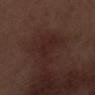The lesion was photographed on a routine skin check and not biopsied; there is no pathology result.
The tile uses white-light illumination.
Automated tile analysis of the lesion measured an area of roughly 6.5 mm². And it measured about 4 CIELAB-L* units darker than the surrounding skin and a normalized border contrast of about 5. And it measured a border-irregularity index near 5/10 and internal color variation of about 2 on a 0–10 scale. It also reported an automated nevus-likeness rating near 0 out of 100 and a detector confidence of about 100 out of 100 that the crop contains a lesion.
The lesion's longest dimension is about 4 mm.
A 15 mm close-up tile from a total-body photography series done for melanoma screening.
The lesion is on the left lower leg.
A male subject, about 70 years old.